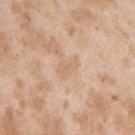Impression: No biopsy was performed on this lesion — it was imaged during a full skin examination and was not determined to be concerning. Acquisition and patient details: Automated tile analysis of the lesion measured a shape eccentricity near 0.85. Longest diameter approximately 2.5 mm. A lesion tile, about 15 mm wide, cut from a 3D total-body photograph. On the arm. The patient is a female about 25 years old.